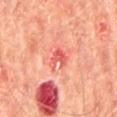Recorded during total-body skin imaging; not selected for excision or biopsy. This is a cross-polarized tile. The subject is a male roughly 65 years of age. The recorded lesion diameter is about 3 mm. The lesion is located on the mid back. This image is a 15 mm lesion crop taken from a total-body photograph.